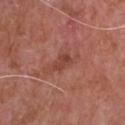{
  "biopsy_status": "not biopsied; imaged during a skin examination",
  "patient": {
    "sex": "male",
    "age_approx": 75
  },
  "site": "chest",
  "image": {
    "source": "total-body photography crop",
    "field_of_view_mm": 15
  },
  "lesion_size": {
    "long_diameter_mm_approx": 2.5
  }
}